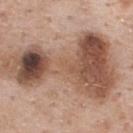| field | value |
|---|---|
| notes | catalogued during a skin exam; not biopsied |
| patient | male, in their 60s |
| body site | the upper back |
| lesion diameter | about 14.5 mm |
| imaging modality | ~15 mm tile from a whole-body skin photo |